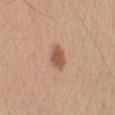Captured during whole-body skin photography for melanoma surveillance; the lesion was not biopsied. A close-up tile cropped from a whole-body skin photograph, about 15 mm across. Located on the left upper arm. A male patient, aged around 55. Imaged with white-light lighting. The lesion-visualizer software estimated an area of roughly 5 mm², an eccentricity of roughly 0.65, and a symmetry-axis asymmetry near 0.25. It also reported a mean CIELAB color near L≈55 a*≈22 b*≈32, about 12 CIELAB-L* units darker than the surrounding skin, and a normalized lesion–skin contrast near 8.5. And it measured a classifier nevus-likeness of about 95/100 and lesion-presence confidence of about 100/100. The recorded lesion diameter is about 3 mm.